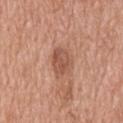| feature | finding |
|---|---|
| acquisition | ~15 mm tile from a whole-body skin photo |
| size | about 3.5 mm |
| TBP lesion metrics | a lesion area of about 6.5 mm² |
| illumination | white-light |
| site | the upper back |
| patient | male, in their mid-50s |